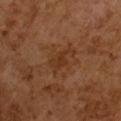| field | value |
|---|---|
| image | ~15 mm crop, total-body skin-cancer survey |
| subject | male, aged 63–67 |